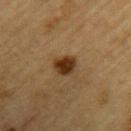{"biopsy_status": "not biopsied; imaged during a skin examination", "image": {"source": "total-body photography crop", "field_of_view_mm": 15}, "patient": {"sex": "male", "age_approx": "85+"}, "automated_metrics": {"cielab_L": 29, "cielab_a": 16, "cielab_b": 29, "vs_skin_darker_L": 12.0, "vs_skin_contrast_norm": 12.0, "color_variation_0_10": 2.5}, "lesion_size": {"long_diameter_mm_approx": 3.0}, "site": "left upper arm"}